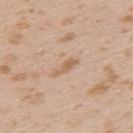This lesion was catalogued during total-body skin photography and was not selected for biopsy.
Captured under white-light illumination.
The patient is a female aged 23–27.
Located on the left upper arm.
About 3 mm across.
A 15 mm crop from a total-body photograph taken for skin-cancer surveillance.